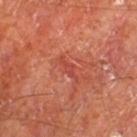  biopsy_status: not biopsied; imaged during a skin examination
  patient:
    sex: male
    age_approx: 65
  automated_metrics:
    cielab_L: 45
    cielab_a: 34
    cielab_b: 32
    vs_skin_darker_L: 6.0
    vs_skin_contrast_norm: 5.0
    color_variation_0_10: 0.0
    peripheral_color_asymmetry: 0.0
    nevus_likeness_0_100: 0
    lesion_detection_confidence_0_100: 95
  site: left thigh
  image:
    source: total-body photography crop
    field_of_view_mm: 15